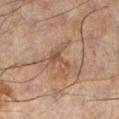Clinical impression: No biopsy was performed on this lesion — it was imaged during a full skin examination and was not determined to be concerning.